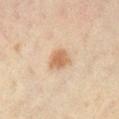Captured during whole-body skin photography for melanoma surveillance; the lesion was not biopsied.
A 15 mm close-up extracted from a 3D total-body photography capture.
Located on the right lower leg.
The subject is a female approximately 45 years of age.
Measured at roughly 3 mm in maximum diameter.
An algorithmic analysis of the crop reported a lesion color around L≈55 a*≈17 b*≈31 in CIELAB, about 10 CIELAB-L* units darker than the surrounding skin, and a normalized border contrast of about 8. The software also gave a detector confidence of about 100 out of 100 that the crop contains a lesion.
The tile uses cross-polarized illumination.